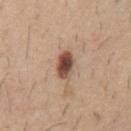Findings:
* follow-up: no biopsy performed (imaged during a skin exam)
* imaging modality: total-body-photography crop, ~15 mm field of view
* patient: male, aged approximately 60
* location: the chest
* illumination: white-light